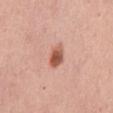The lesion was tiled from a total-body skin photograph and was not biopsied. Measured at roughly 3 mm in maximum diameter. An algorithmic analysis of the crop reported a footprint of about 4.5 mm², an eccentricity of roughly 0.75, and a symmetry-axis asymmetry near 0.2. It also reported about 15 CIELAB-L* units darker than the surrounding skin. Cropped from a whole-body photographic skin survey; the tile spans about 15 mm. Imaged with white-light lighting. From the back. A female patient, aged around 60.